A 15 mm close-up tile from a total-body photography series done for melanoma screening, longest diameter approximately 7.5 mm, captured under white-light illumination, the lesion is located on the front of the torso, the subject is a male roughly 80 years of age, Automated tile analysis of the lesion measured a border-irregularity index near 3.5/10 and a peripheral color-asymmetry measure near 3.5. It also reported a nevus-likeness score of about 5/100 and a detector confidence of about 100 out of 100 that the crop contains a lesion.
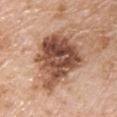histopathologic diagnosis = a melanoma in situ, lentigo maligna type